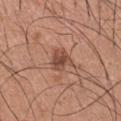Clinical impression: The lesion was photographed on a routine skin check and not biopsied; there is no pathology result. Clinical summary: Captured under white-light illumination. Measured at roughly 3 mm in maximum diameter. On the arm. A male subject, aged 33–37. This image is a 15 mm lesion crop taken from a total-body photograph. Automated tile analysis of the lesion measured a lesion area of about 5.5 mm² and two-axis asymmetry of about 0.3. The analysis additionally found a lesion–skin lightness drop of about 11. The analysis additionally found border irregularity of about 3 on a 0–10 scale, a within-lesion color-variation index near 3.5/10, and peripheral color asymmetry of about 1. And it measured a nevus-likeness score of about 30/100.